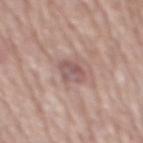Q: Is there a histopathology result?
A: no biopsy performed (imaged during a skin exam)
Q: How large is the lesion?
A: ≈3 mm
Q: What are the patient's age and sex?
A: male, approximately 75 years of age
Q: What is the anatomic site?
A: the mid back
Q: What is the imaging modality?
A: ~15 mm crop, total-body skin-cancer survey
Q: Illumination type?
A: white-light illumination